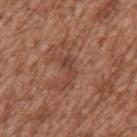Imaged during a routine full-body skin examination; the lesion was not biopsied and no histopathology is available. The subject is a male about 45 years old. From the left upper arm. Imaged with white-light lighting. Longest diameter approximately 3.5 mm. The lesion-visualizer software estimated a lesion area of about 4.5 mm² and a shape eccentricity near 0.85. And it measured about 7 CIELAB-L* units darker than the surrounding skin and a normalized lesion–skin contrast near 6. The software also gave a border-irregularity index near 6/10, a color-variation rating of about 3/10, and radial color variation of about 1.5. The analysis additionally found a classifier nevus-likeness of about 0/100. A lesion tile, about 15 mm wide, cut from a 3D total-body photograph.